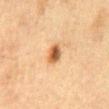{
  "biopsy_status": "not biopsied; imaged during a skin examination"
}